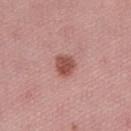Q: Was this lesion biopsied?
A: total-body-photography surveillance lesion; no biopsy
Q: What are the patient's age and sex?
A: female, in their mid- to late 50s
Q: How large is the lesion?
A: ≈3 mm
Q: Where on the body is the lesion?
A: the lower back
Q: What kind of image is this?
A: ~15 mm tile from a whole-body skin photo